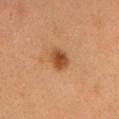The lesion was photographed on a routine skin check and not biopsied; there is no pathology result.
Captured under cross-polarized illumination.
The total-body-photography lesion software estimated an area of roughly 5.5 mm², an eccentricity of roughly 0.7, and two-axis asymmetry of about 0.15. And it measured a mean CIELAB color near L≈44 a*≈23 b*≈35, about 12 CIELAB-L* units darker than the surrounding skin, and a lesion-to-skin contrast of about 9 (normalized; higher = more distinct). And it measured a color-variation rating of about 3/10 and radial color variation of about 1. It also reported a nevus-likeness score of about 95/100 and lesion-presence confidence of about 100/100.
A female subject aged 28 to 32.
The lesion is located on the head or neck.
The lesion's longest dimension is about 3 mm.
This image is a 15 mm lesion crop taken from a total-body photograph.